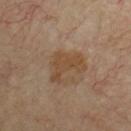Assessment:
Captured during whole-body skin photography for melanoma surveillance; the lesion was not biopsied.
Acquisition and patient details:
The lesion-visualizer software estimated border irregularity of about 6.5 on a 0–10 scale and a peripheral color-asymmetry measure near 0.5. The lesion's longest dimension is about 4.5 mm. The tile uses cross-polarized illumination. A close-up tile cropped from a whole-body skin photograph, about 15 mm across. A male patient about 65 years old. The lesion is located on the chest.